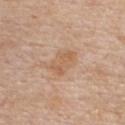{
  "biopsy_status": "not biopsied; imaged during a skin examination",
  "image": {
    "source": "total-body photography crop",
    "field_of_view_mm": 15
  },
  "patient": {
    "sex": "male",
    "age_approx": 60
  },
  "site": "chest"
}